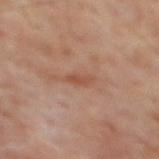Case summary:
– notes — imaged on a skin check; not biopsied
– patient — male, aged 63–67
– acquisition — ~15 mm tile from a whole-body skin photo
– location — the mid back